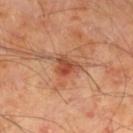This lesion was catalogued during total-body skin photography and was not selected for biopsy.
A region of skin cropped from a whole-body photographic capture, roughly 15 mm wide.
Captured under cross-polarized illumination.
The subject is a male about 70 years old.
Approximately 3.5 mm at its widest.
Located on the right lower leg.
The total-body-photography lesion software estimated border irregularity of about 3.5 on a 0–10 scale, internal color variation of about 7 on a 0–10 scale, and a peripheral color-asymmetry measure near 3. And it measured a detector confidence of about 100 out of 100 that the crop contains a lesion.